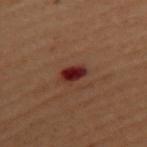A region of skin cropped from a whole-body photographic capture, roughly 15 mm wide.
The tile uses cross-polarized illumination.
A female patient approximately 50 years of age.
Measured at roughly 3 mm in maximum diameter.
The lesion is located on the back.